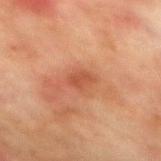The lesion was tiled from a total-body skin photograph and was not biopsied.